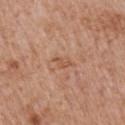No biopsy was performed on this lesion — it was imaged during a full skin examination and was not determined to be concerning.